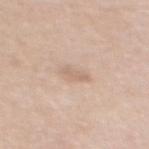Part of a total-body skin-imaging series; this lesion was reviewed on a skin check and was not flagged for biopsy. The patient is a female roughly 40 years of age. Measured at roughly 2.5 mm in maximum diameter. Imaged with white-light lighting. This image is a 15 mm lesion crop taken from a total-body photograph. The total-body-photography lesion software estimated a mean CIELAB color near L≈65 a*≈16 b*≈28, a lesion–skin lightness drop of about 7, and a lesion-to-skin contrast of about 4.5 (normalized; higher = more distinct). The analysis additionally found a border-irregularity rating of about 3/10 and a within-lesion color-variation index near 0.5/10. From the back.